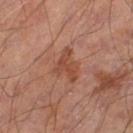| key | value |
|---|---|
| follow-up | total-body-photography surveillance lesion; no biopsy |
| TBP lesion metrics | a footprint of about 8.5 mm², an eccentricity of roughly 0.65, and two-axis asymmetry of about 0.45; a mean CIELAB color near L≈44 a*≈22 b*≈29, a lesion–skin lightness drop of about 7, and a normalized border contrast of about 6.5; a border-irregularity index near 5/10, a within-lesion color-variation index near 3/10, and radial color variation of about 1 |
| image | ~15 mm tile from a whole-body skin photo |
| illumination | cross-polarized illumination |
| subject | male, aged 53–57 |
| anatomic site | the left thigh |
| lesion size | about 4 mm |